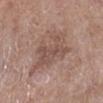Notes:
* follow-up — catalogued during a skin exam; not biopsied
* TBP lesion metrics — a color-variation rating of about 3/10 and peripheral color asymmetry of about 1
* lesion size — about 6 mm
* image source — 15 mm crop, total-body photography
* illumination — white-light illumination
* subject — male, about 50 years old
* body site — the right lower leg